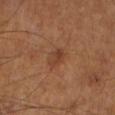Clinical impression:
The lesion was photographed on a routine skin check and not biopsied; there is no pathology result.
Image and clinical context:
Imaged with cross-polarized lighting. A 15 mm crop from a total-body photograph taken for skin-cancer surveillance. Located on the left leg. Longest diameter approximately 2.5 mm. Automated image analysis of the tile measured an area of roughly 3 mm², an eccentricity of roughly 0.8, and a symmetry-axis asymmetry near 0.35. The analysis additionally found an average lesion color of about L≈38 a*≈21 b*≈30 (CIELAB), roughly 7 lightness units darker than nearby skin, and a lesion-to-skin contrast of about 6 (normalized; higher = more distinct). And it measured a border-irregularity rating of about 3.5/10, a color-variation rating of about 1.5/10, and radial color variation of about 0.5. The analysis additionally found a lesion-detection confidence of about 100/100. A male subject, aged 63 to 67.